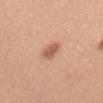Part of a total-body skin-imaging series; this lesion was reviewed on a skin check and was not flagged for biopsy. Measured at roughly 2.5 mm in maximum diameter. Imaged with white-light lighting. Located on the abdomen. A female subject, aged 28–32. A roughly 15 mm field-of-view crop from a total-body skin photograph.